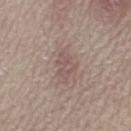The lesion's longest dimension is about 3 mm. The lesion is on the leg. Imaged with white-light lighting. A female subject aged 58–62. This image is a 15 mm lesion crop taken from a total-body photograph.